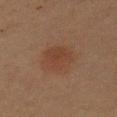follow-up = total-body-photography surveillance lesion; no biopsy | patient = female, approximately 60 years of age | body site = the left upper arm | lesion diameter = about 4 mm | tile lighting = cross-polarized | image = ~15 mm crop, total-body skin-cancer survey.